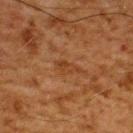Imaged during a routine full-body skin examination; the lesion was not biopsied and no histopathology is available.
This is a cross-polarized tile.
A 15 mm close-up extracted from a 3D total-body photography capture.
The lesion is located on the upper back.
Automated image analysis of the tile measured a mean CIELAB color near L≈34 a*≈21 b*≈32, a lesion–skin lightness drop of about 6, and a lesion-to-skin contrast of about 6 (normalized; higher = more distinct). It also reported a nevus-likeness score of about 0/100.
About 3 mm across.
A male patient, aged approximately 60.